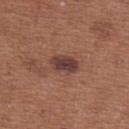Clinical summary:
A 15 mm close-up extracted from a 3D total-body photography capture. The lesion's longest dimension is about 4 mm. From the upper back. A female subject aged around 65. The total-body-photography lesion software estimated a lesion area of about 6.5 mm² and a shape eccentricity near 0.85. The analysis additionally found a nevus-likeness score of about 30/100. The tile uses white-light illumination.